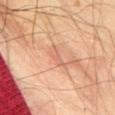| key | value |
|---|---|
| notes | total-body-photography surveillance lesion; no biopsy |
| patient | male, aged 63 to 67 |
| imaging modality | ~15 mm crop, total-body skin-cancer survey |